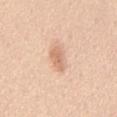follow-up = imaged on a skin check; not biopsied | image = 15 mm crop, total-body photography | site = the chest | patient = male, aged 43 to 47 | diameter = ≈3.5 mm | lighting = white-light illumination.